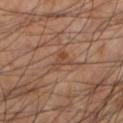Impression: Part of a total-body skin-imaging series; this lesion was reviewed on a skin check and was not flagged for biopsy. Acquisition and patient details: The patient is a male aged around 60. On the left lower leg. This is a cross-polarized tile. An algorithmic analysis of the crop reported a shape eccentricity near 0.65 and a symmetry-axis asymmetry near 0.45. It also reported border irregularity of about 4 on a 0–10 scale, a within-lesion color-variation index near 4/10, and radial color variation of about 1. A region of skin cropped from a whole-body photographic capture, roughly 15 mm wide.